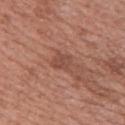Clinical impression: The lesion was tiled from a total-body skin photograph and was not biopsied. Acquisition and patient details: A female patient aged 38 to 42. This image is a 15 mm lesion crop taken from a total-body photograph. On the upper back.